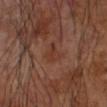biopsy_status: not biopsied; imaged during a skin examination
lighting: cross-polarized
site: arm
image:
  source: total-body photography crop
  field_of_view_mm: 15
lesion_size:
  long_diameter_mm_approx: 2.5
patient:
  sex: male
  age_approx: 70
automated_metrics:
  area_mm2_approx: 3.5
  eccentricity: 0.6
  shape_asymmetry: 0.55
  vs_skin_darker_L: 5.0
  vs_skin_contrast_norm: 5.5
  border_irregularity_0_10: 5.0
  peripheral_color_asymmetry: 0.5
  nevus_likeness_0_100: 0
  lesion_detection_confidence_0_100: 100